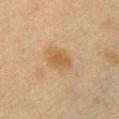The patient is a female approximately 40 years of age. A lesion tile, about 15 mm wide, cut from a 3D total-body photograph. The lesion is located on the chest.